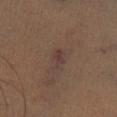follow-up: catalogued during a skin exam; not biopsied | TBP lesion metrics: an eccentricity of roughly 0.85; a detector confidence of about 95 out of 100 that the crop contains a lesion | size: ≈3 mm | site: the leg | subject: male, in their mid-40s | lighting: cross-polarized illumination | image: ~15 mm crop, total-body skin-cancer survey.